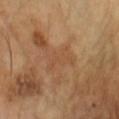Q: Was a biopsy performed?
A: total-body-photography surveillance lesion; no biopsy
Q: Where on the body is the lesion?
A: the head or neck
Q: What kind of image is this?
A: ~15 mm tile from a whole-body skin photo
Q: Illumination type?
A: cross-polarized
Q: What are the patient's age and sex?
A: male, in their mid- to late 40s
Q: How large is the lesion?
A: about 3.5 mm